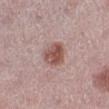follow-up: imaged on a skin check; not biopsied
imaging modality: ~15 mm tile from a whole-body skin photo
diameter: about 3.5 mm
patient: female, approximately 45 years of age
lighting: white-light illumination
site: the leg
image-analysis metrics: a mean CIELAB color near L≈52 a*≈21 b*≈22, a lesion–skin lightness drop of about 12, and a normalized lesion–skin contrast near 8.5; border irregularity of about 2 on a 0–10 scale, a color-variation rating of about 5/10, and a peripheral color-asymmetry measure near 1.5; a nevus-likeness score of about 85/100 and lesion-presence confidence of about 100/100